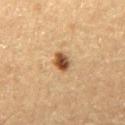Findings:
– follow-up: catalogued during a skin exam; not biopsied
– lesion size: about 2.5 mm
– subject: male, aged around 60
– acquisition: 15 mm crop, total-body photography
– illumination: cross-polarized